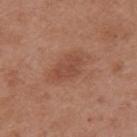Recorded during total-body skin imaging; not selected for excision or biopsy. Measured at roughly 4 mm in maximum diameter. The lesion is on the upper back. A female subject, about 40 years old. Cropped from a whole-body photographic skin survey; the tile spans about 15 mm. Captured under white-light illumination.